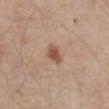A 15 mm crop from a total-body photograph taken for skin-cancer surveillance. The lesion is on the front of the torso. The subject is a male aged around 80. Captured under white-light illumination. About 2.5 mm across.